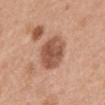No biopsy was performed on this lesion — it was imaged during a full skin examination and was not determined to be concerning.
The lesion's longest dimension is about 4.5 mm.
A male subject, aged 28 to 32.
The tile uses white-light illumination.
A 15 mm close-up tile from a total-body photography series done for melanoma screening.
The lesion is located on the mid back.